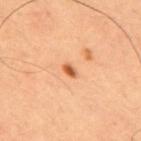biopsy_status: not biopsied; imaged during a skin examination
patient:
  sex: male
  age_approx: 65
image:
  source: total-body photography crop
  field_of_view_mm: 15
site: back
lesion_size:
  long_diameter_mm_approx: 2.0